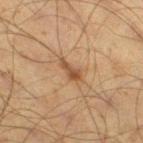{"site": "right thigh", "patient": {"sex": "male", "age_approx": 60}, "image": {"source": "total-body photography crop", "field_of_view_mm": 15}}